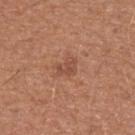biopsy_status: not biopsied; imaged during a skin examination
site: left upper arm
lighting: white-light
patient:
  sex: male
  age_approx: 55
lesion_size:
  long_diameter_mm_approx: 3.0
image:
  source: total-body photography crop
  field_of_view_mm: 15